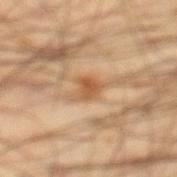{"biopsy_status": "not biopsied; imaged during a skin examination", "site": "left lower leg", "image": {"source": "total-body photography crop", "field_of_view_mm": 15}, "lesion_size": {"long_diameter_mm_approx": 2.5}, "patient": {"sex": "male", "age_approx": 45}, "lighting": "cross-polarized"}